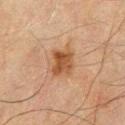The lesion was tiled from a total-body skin photograph and was not biopsied. A region of skin cropped from a whole-body photographic capture, roughly 15 mm wide. Measured at roughly 3.5 mm in maximum diameter. The patient is a male aged 68 to 72. On the front of the torso.